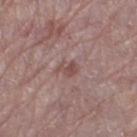This lesion was catalogued during total-body skin photography and was not selected for biopsy.
Automated tile analysis of the lesion measured a lesion area of about 3.5 mm² and an outline eccentricity of about 0.65 (0 = round, 1 = elongated). And it measured a lesion-detection confidence of about 100/100.
Cropped from a whole-body photographic skin survey; the tile spans about 15 mm.
Imaged with white-light lighting.
A female patient, in their mid-60s.
The lesion is on the left thigh.